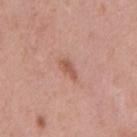Q: Was this lesion biopsied?
A: total-body-photography surveillance lesion; no biopsy
Q: What is the imaging modality?
A: ~15 mm crop, total-body skin-cancer survey
Q: Who is the patient?
A: male, aged around 40
Q: What is the anatomic site?
A: the back